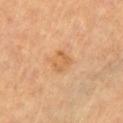This lesion was catalogued during total-body skin photography and was not selected for biopsy. Measured at roughly 3 mm in maximum diameter. A male patient, aged 83 or over. The total-body-photography lesion software estimated an area of roughly 6 mm², a shape eccentricity near 0.45, and two-axis asymmetry of about 0.2. And it measured border irregularity of about 2 on a 0–10 scale and a within-lesion color-variation index near 3.5/10. It also reported an automated nevus-likeness rating near 10 out of 100 and lesion-presence confidence of about 100/100. The lesion is located on the chest. Cropped from a total-body skin-imaging series; the visible field is about 15 mm. The tile uses cross-polarized illumination.